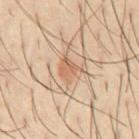follow-up: total-body-photography surveillance lesion; no biopsy.